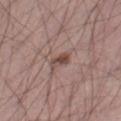Findings:
– illumination: white-light
– size: about 3 mm
– image source: ~15 mm tile from a whole-body skin photo
– site: the right thigh
– patient: male, aged 53 to 57